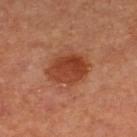Recorded during total-body skin imaging; not selected for excision or biopsy. Automated tile analysis of the lesion measured an area of roughly 13 mm² and a shape-asymmetry score of about 0.15 (0 = symmetric). It also reported about 11 CIELAB-L* units darker than the surrounding skin and a normalized border contrast of about 9. The analysis additionally found a classifier nevus-likeness of about 100/100 and a lesion-detection confidence of about 100/100. The lesion's longest dimension is about 5 mm. Captured under cross-polarized illumination. A 15 mm crop from a total-body photograph taken for skin-cancer surveillance. The lesion is on the right lower leg. A female patient.